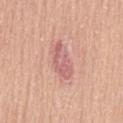<case>
<biopsy_status>not biopsied; imaged during a skin examination</biopsy_status>
<site>lower back</site>
<patient>
  <sex>female</sex>
  <age_approx>65</age_approx>
</patient>
<image>
  <source>total-body photography crop</source>
  <field_of_view_mm>15</field_of_view_mm>
</image>
</case>